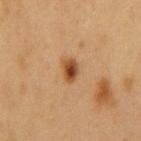* biopsy status: catalogued during a skin exam; not biopsied
* image-analysis metrics: a nevus-likeness score of about 100/100 and a detector confidence of about 100 out of 100 that the crop contains a lesion
* illumination: cross-polarized
* diameter: ~2.5 mm (longest diameter)
* body site: the front of the torso
* image: 15 mm crop, total-body photography
* subject: male, approximately 55 years of age